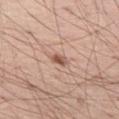workup: total-body-photography surveillance lesion; no biopsy | location: the left thigh | patient: male, about 55 years old | automated lesion analysis: a footprint of about 3 mm², a shape eccentricity near 0.85, and a shape-asymmetry score of about 0.25 (0 = symmetric); a mean CIELAB color near L≈56 a*≈21 b*≈28, about 12 CIELAB-L* units darker than the surrounding skin, and a normalized lesion–skin contrast near 8; border irregularity of about 2.5 on a 0–10 scale and internal color variation of about 3 on a 0–10 scale; a nevus-likeness score of about 85/100 | lesion diameter: ~2.5 mm (longest diameter) | illumination: white-light | imaging modality: ~15 mm crop, total-body skin-cancer survey.